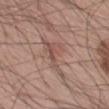| feature | finding |
|---|---|
| follow-up | no biopsy performed (imaged during a skin exam) |
| site | the leg |
| lesion size | ~6.5 mm (longest diameter) |
| patient | male, roughly 60 years of age |
| image source | ~15 mm tile from a whole-body skin photo |
| TBP lesion metrics | a mean CIELAB color near L≈52 a*≈19 b*≈24, roughly 8 lightness units darker than nearby skin, and a normalized border contrast of about 5.5; a border-irregularity index near 8.5/10, a color-variation rating of about 5.5/10, and radial color variation of about 2; an automated nevus-likeness rating near 0 out of 100 and lesion-presence confidence of about 50/100 |
| tile lighting | white-light illumination |